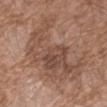subject: male, in their 50s
anatomic site: the right forearm
imaging modality: ~15 mm tile from a whole-body skin photo